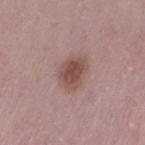– workup: total-body-photography surveillance lesion; no biopsy
– imaging modality: 15 mm crop, total-body photography
– location: the left thigh
– image-analysis metrics: internal color variation of about 3.5 on a 0–10 scale and a peripheral color-asymmetry measure near 1; a classifier nevus-likeness of about 95/100
– lighting: white-light
– patient: female, aged around 50